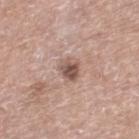Assessment: Recorded during total-body skin imaging; not selected for excision or biopsy. Image and clinical context: A male subject aged 78–82. The tile uses white-light illumination. A 15 mm crop from a total-body photograph taken for skin-cancer surveillance. On the right lower leg.